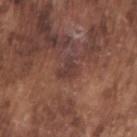• follow-up · total-body-photography surveillance lesion; no biopsy
• image-analysis metrics · a shape-asymmetry score of about 0.45 (0 = symmetric)
• subject · male, in their mid-70s
• image · 15 mm crop, total-body photography
• anatomic site · the right upper arm
• lesion diameter · about 2.5 mm